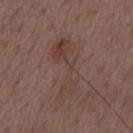biopsy status: imaged on a skin check; not biopsied | patient: male, approximately 50 years of age | tile lighting: white-light | size: ~8 mm (longest diameter) | anatomic site: the mid back | acquisition: ~15 mm tile from a whole-body skin photo.